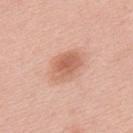Q: Was this lesion biopsied?
A: total-body-photography surveillance lesion; no biopsy
Q: What is the anatomic site?
A: the upper back
Q: How was this image acquired?
A: ~15 mm crop, total-body skin-cancer survey
Q: How was the tile lit?
A: white-light
Q: Patient demographics?
A: male, aged around 55